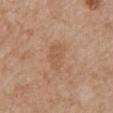Notes:
- image source — total-body-photography crop, ~15 mm field of view
- patient — female, approximately 70 years of age
- body site — the chest
- size — ~3.5 mm (longest diameter)
- TBP lesion metrics — a lesion area of about 5.5 mm² and an eccentricity of roughly 0.85; a lesion color around L≈55 a*≈20 b*≈33 in CIELAB, a lesion–skin lightness drop of about 6, and a normalized lesion–skin contrast near 5